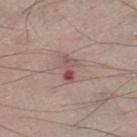workup = catalogued during a skin exam; not biopsied
location = the right lower leg
subject = male, roughly 55 years of age
size = ≈3.5 mm
acquisition = ~15 mm crop, total-body skin-cancer survey
tile lighting = white-light